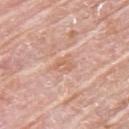biopsy status: catalogued during a skin exam; not biopsied | automated metrics: a footprint of about 4 mm² and an outline eccentricity of about 0.7 (0 = round, 1 = elongated) | image: total-body-photography crop, ~15 mm field of view | subject: female, in their mid-60s | diameter: ~2.5 mm (longest diameter) | illumination: white-light | anatomic site: the upper back.